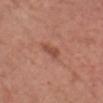The lesion was photographed on a routine skin check and not biopsied; there is no pathology result.
The lesion-visualizer software estimated a lesion color around L≈49 a*≈25 b*≈29 in CIELAB, roughly 9 lightness units darker than nearby skin, and a normalized lesion–skin contrast near 6.5. And it measured an automated nevus-likeness rating near 20 out of 100 and lesion-presence confidence of about 100/100.
A male subject, aged 58 to 62.
A 15 mm close-up extracted from a 3D total-body photography capture.
On the head or neck.